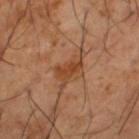Assessment:
Recorded during total-body skin imaging; not selected for excision or biopsy.
Image and clinical context:
The lesion is located on the left thigh. A 15 mm close-up extracted from a 3D total-body photography capture. A male subject, roughly 65 years of age.